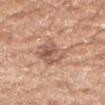Q: Was a biopsy performed?
A: no biopsy performed (imaged during a skin exam)
Q: Patient demographics?
A: female, roughly 75 years of age
Q: What is the lesion's diameter?
A: ~5.5 mm (longest diameter)
Q: What is the anatomic site?
A: the right forearm
Q: How was this image acquired?
A: 15 mm crop, total-body photography